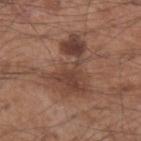workup = catalogued during a skin exam; not biopsied | image = 15 mm crop, total-body photography | size = ~7.5 mm (longest diameter) | site = the left forearm | subject = male, aged approximately 55.